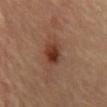The lesion was tiled from a total-body skin photograph and was not biopsied. A male subject roughly 85 years of age or older. A region of skin cropped from a whole-body photographic capture, roughly 15 mm wide. The lesion's longest dimension is about 3.5 mm. An algorithmic analysis of the crop reported a color-variation rating of about 6/10 and a peripheral color-asymmetry measure near 2. And it measured a classifier nevus-likeness of about 90/100. Captured under cross-polarized illumination. The lesion is on the front of the torso.